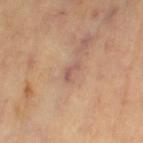workup = imaged on a skin check; not biopsied